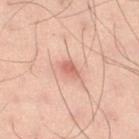{"biopsy_status": "not biopsied; imaged during a skin examination"}